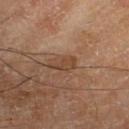Q: Is there a histopathology result?
A: imaged on a skin check; not biopsied
Q: Where on the body is the lesion?
A: the right lower leg
Q: How was this image acquired?
A: 15 mm crop, total-body photography
Q: What are the patient's age and sex?
A: male, aged around 65